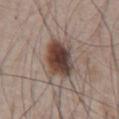{
  "biopsy_status": "not biopsied; imaged during a skin examination",
  "site": "chest",
  "patient": {
    "sex": "male",
    "age_approx": 65
  },
  "image": {
    "source": "total-body photography crop",
    "field_of_view_mm": 15
  }
}